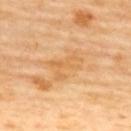workup: imaged on a skin check; not biopsied
image-analysis metrics: a lesion area of about 7.5 mm², an outline eccentricity of about 0.85 (0 = round, 1 = elongated), and two-axis asymmetry of about 0.5; a border-irregularity index near 6.5/10 and internal color variation of about 3 on a 0–10 scale; a nevus-likeness score of about 0/100
subject: female, aged around 60
site: the upper back
illumination: cross-polarized illumination
lesion size: ~4 mm (longest diameter)
image source: 15 mm crop, total-body photography